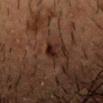No biopsy was performed on this lesion — it was imaged during a full skin examination and was not determined to be concerning. A male subject aged 48–52. Automated image analysis of the tile measured an area of roughly 3 mm², an eccentricity of roughly 0.95, and a shape-asymmetry score of about 0.4 (0 = symmetric). And it measured an average lesion color of about L≈15 a*≈15 b*≈17 (CIELAB), a lesion–skin lightness drop of about 8, and a normalized border contrast of about 10.5. The software also gave a border-irregularity rating of about 4/10, internal color variation of about 0 on a 0–10 scale, and radial color variation of about 0. The software also gave a nevus-likeness score of about 0/100 and a detector confidence of about 90 out of 100 that the crop contains a lesion. From the head or neck. Approximately 3.5 mm at its widest. Imaged with cross-polarized lighting. A lesion tile, about 15 mm wide, cut from a 3D total-body photograph.